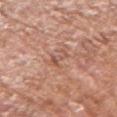Clinical impression:
Part of a total-body skin-imaging series; this lesion was reviewed on a skin check and was not flagged for biopsy.